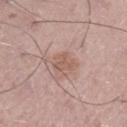{"image": {"source": "total-body photography crop", "field_of_view_mm": 15}, "patient": {"sex": "male", "age_approx": 50}, "lesion_size": {"long_diameter_mm_approx": 3.0}, "site": "right lower leg", "lighting": "white-light"}